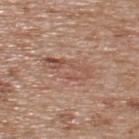This lesion was catalogued during total-body skin photography and was not selected for biopsy. This is a white-light tile. A lesion tile, about 15 mm wide, cut from a 3D total-body photograph. The lesion is located on the upper back. A male subject, about 65 years old.